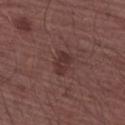Part of a total-body skin-imaging series; this lesion was reviewed on a skin check and was not flagged for biopsy. A close-up tile cropped from a whole-body skin photograph, about 15 mm across. This is a white-light tile. About 3 mm across. An algorithmic analysis of the crop reported a lesion area of about 5.5 mm², an eccentricity of roughly 0.7, and a symmetry-axis asymmetry near 0.25. The software also gave a within-lesion color-variation index near 2/10 and radial color variation of about 0.5. And it measured a nevus-likeness score of about 10/100 and lesion-presence confidence of about 100/100. A male patient, aged around 65. Located on the right upper arm.